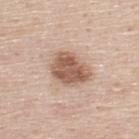No biopsy was performed on this lesion — it was imaged during a full skin examination and was not determined to be concerning. A 15 mm close-up tile from a total-body photography series done for melanoma screening. A male patient roughly 45 years of age. Approximately 4.5 mm at its widest. The lesion is on the upper back. The tile uses white-light illumination.